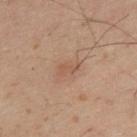Q: How was the tile lit?
A: cross-polarized
Q: What is the lesion's diameter?
A: about 2.5 mm
Q: How was this image acquired?
A: total-body-photography crop, ~15 mm field of view
Q: Who is the patient?
A: male, in their 50s
Q: What is the anatomic site?
A: the back